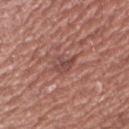Q: Was a biopsy performed?
A: no biopsy performed (imaged during a skin exam)
Q: What lighting was used for the tile?
A: white-light illumination
Q: What did automated image analysis measure?
A: a lesion color around L≈46 a*≈23 b*≈24 in CIELAB, roughly 9 lightness units darker than nearby skin, and a normalized border contrast of about 6.5
Q: How was this image acquired?
A: 15 mm crop, total-body photography
Q: Who is the patient?
A: male, roughly 45 years of age
Q: Lesion location?
A: the left upper arm
Q: Lesion size?
A: about 2.5 mm